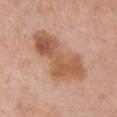Clinical impression:
The lesion was tiled from a total-body skin photograph and was not biopsied.
Clinical summary:
A female subject roughly 65 years of age. The lesion is located on the chest. A 15 mm crop from a total-body photograph taken for skin-cancer surveillance. An algorithmic analysis of the crop reported a footprint of about 25 mm² and an eccentricity of roughly 0.9. And it measured roughly 11 lightness units darker than nearby skin and a lesion-to-skin contrast of about 8 (normalized; higher = more distinct). And it measured a border-irregularity index near 4.5/10, a within-lesion color-variation index near 5.5/10, and radial color variation of about 2. The analysis additionally found a nevus-likeness score of about 0/100. Measured at roughly 8 mm in maximum diameter. The tile uses white-light illumination.